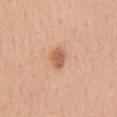Q: Was a biopsy performed?
A: total-body-photography surveillance lesion; no biopsy
Q: Patient demographics?
A: female, approximately 50 years of age
Q: What did automated image analysis measure?
A: an average lesion color of about L≈61 a*≈24 b*≈34 (CIELAB) and a lesion–skin lightness drop of about 11; a color-variation rating of about 2/10 and a peripheral color-asymmetry measure near 1; a detector confidence of about 100 out of 100 that the crop contains a lesion
Q: What is the lesion's diameter?
A: ≈3 mm
Q: What lighting was used for the tile?
A: white-light illumination
Q: What is the anatomic site?
A: the back
Q: What is the imaging modality?
A: 15 mm crop, total-body photography